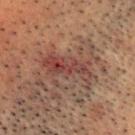Imaged during a routine full-body skin examination; the lesion was not biopsied and no histopathology is available.
Cropped from a total-body skin-imaging series; the visible field is about 15 mm.
A male patient roughly 55 years of age.
From the head or neck.